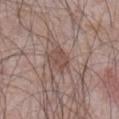patient: male, in their mid- to late 60s
automated metrics: a lesion area of about 6 mm², an outline eccentricity of about 0.65 (0 = round, 1 = elongated), and a symmetry-axis asymmetry near 0.3
image: ~15 mm crop, total-body skin-cancer survey
location: the abdomen
illumination: white-light illumination
lesion diameter: ≈3 mm